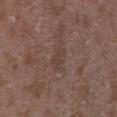{
  "biopsy_status": "not biopsied; imaged during a skin examination",
  "site": "arm",
  "image": {
    "source": "total-body photography crop",
    "field_of_view_mm": 15
  },
  "patient": {
    "sex": "female",
    "age_approx": 35
  }
}